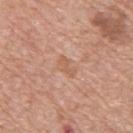  biopsy_status: not biopsied; imaged during a skin examination
  image:
    source: total-body photography crop
    field_of_view_mm: 15
  site: upper back
  lesion_size:
    long_diameter_mm_approx: 2.5
  patient:
    sex: male
    age_approx: 60
  automated_metrics:
    nevus_likeness_0_100: 0
    lesion_detection_confidence_0_100: 100
  lighting: white-light